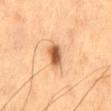The lesion was photographed on a routine skin check and not biopsied; there is no pathology result.
Located on the mid back.
Approximately 3 mm at its widest.
A male subject, approximately 60 years of age.
A 15 mm crop from a total-body photograph taken for skin-cancer surveillance.
This is a cross-polarized tile.